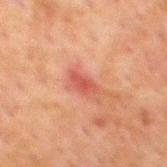A roughly 15 mm field-of-view crop from a total-body skin photograph.
The lesion is located on the back.
A male patient aged 58 to 62.